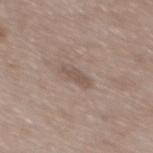Part of a total-body skin-imaging series; this lesion was reviewed on a skin check and was not flagged for biopsy. A 15 mm close-up extracted from a 3D total-body photography capture. The recorded lesion diameter is about 3 mm. The tile uses white-light illumination. A male patient, aged around 50. Located on the mid back.